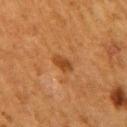{"biopsy_status": "not biopsied; imaged during a skin examination", "image": {"source": "total-body photography crop", "field_of_view_mm": 15}, "patient": {"sex": "female", "age_approx": 50}, "lighting": "cross-polarized", "site": "right upper arm", "lesion_size": {"long_diameter_mm_approx": 2.5}}